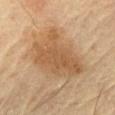{"biopsy_status": "not biopsied; imaged during a skin examination", "lighting": "cross-polarized", "automated_metrics": {"area_mm2_approx": 27.0, "eccentricity": 0.6, "shape_asymmetry": 0.3, "cielab_L": 50, "cielab_a": 17, "cielab_b": 33, "vs_skin_contrast_norm": 7.0}, "image": {"source": "total-body photography crop", "field_of_view_mm": 15}, "site": "chest", "patient": {"sex": "male", "age_approx": 65}, "lesion_size": {"long_diameter_mm_approx": 6.5}}